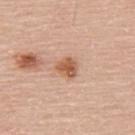Assessment:
No biopsy was performed on this lesion — it was imaged during a full skin examination and was not determined to be concerning.
Clinical summary:
An algorithmic analysis of the crop reported a nevus-likeness score of about 85/100 and a lesion-detection confidence of about 100/100. This is a white-light tile. The lesion is located on the upper back. A 15 mm close-up extracted from a 3D total-body photography capture. A male patient, roughly 60 years of age. The lesion's longest dimension is about 2.5 mm.